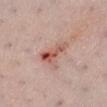biopsy_status: not biopsied; imaged during a skin examination
image:
  source: total-body photography crop
  field_of_view_mm: 15
patient:
  sex: female
  age_approx: 35
lesion_size:
  long_diameter_mm_approx: 5.0
site: leg
automated_metrics:
  cielab_L: 55
  cielab_a: 26
  cielab_b: 27
  vs_skin_darker_L: 12.0
  vs_skin_contrast_norm: 8.5
  border_irregularity_0_10: 5.0
  color_variation_0_10: 9.0
  peripheral_color_asymmetry: 3.0
lighting: white-light